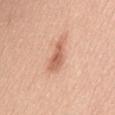Clinical impression:
The lesion was tiled from a total-body skin photograph and was not biopsied.
Background:
The lesion is on the mid back. The subject is a female aged around 45. The lesion-visualizer software estimated a symmetry-axis asymmetry near 0.35. The analysis additionally found a lesion color around L≈61 a*≈25 b*≈33 in CIELAB and a normalized lesion–skin contrast near 7.5. The analysis additionally found a border-irregularity index near 4.5/10, a within-lesion color-variation index near 1.5/10, and a peripheral color-asymmetry measure near 0.5. And it measured a nevus-likeness score of about 60/100 and a detector confidence of about 100 out of 100 that the crop contains a lesion. The lesion's longest dimension is about 4.5 mm. Captured under white-light illumination. A roughly 15 mm field-of-view crop from a total-body skin photograph.